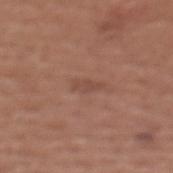Notes:
• notes · no biopsy performed (imaged during a skin exam)
• image source · 15 mm crop, total-body photography
• size · ~3 mm (longest diameter)
• location · the front of the torso
• illumination · white-light
• patient · female, roughly 35 years of age